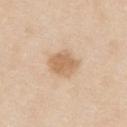follow-up: catalogued during a skin exam; not biopsied
image-analysis metrics: an area of roughly 8.5 mm², an outline eccentricity of about 0.75 (0 = round, 1 = elongated), and a shape-asymmetry score of about 0.2 (0 = symmetric); about 11 CIELAB-L* units darker than the surrounding skin and a lesion-to-skin contrast of about 7 (normalized; higher = more distinct); a border-irregularity index near 2/10, a color-variation rating of about 2/10, and peripheral color asymmetry of about 0.5
site: the upper back
image: total-body-photography crop, ~15 mm field of view
lesion size: ~4 mm (longest diameter)
patient: male, approximately 35 years of age